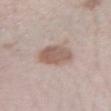Impression: Imaged during a routine full-body skin examination; the lesion was not biopsied and no histopathology is available. Acquisition and patient details: The tile uses white-light illumination. A 15 mm crop from a total-body photograph taken for skin-cancer surveillance. On the left forearm. The patient is a female aged 28–32. About 4 mm across.